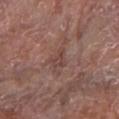Q: What is the lesion's diameter?
A: ~2.5 mm (longest diameter)
Q: How was the tile lit?
A: white-light
Q: Automated lesion metrics?
A: a lesion color around L≈43 a*≈20 b*≈23 in CIELAB and a lesion–skin lightness drop of about 7; border irregularity of about 5 on a 0–10 scale, a within-lesion color-variation index near 1/10, and radial color variation of about 0; a classifier nevus-likeness of about 0/100 and a detector confidence of about 90 out of 100 that the crop contains a lesion
Q: What are the patient's age and sex?
A: male, aged approximately 80
Q: How was this image acquired?
A: total-body-photography crop, ~15 mm field of view
Q: Lesion location?
A: the arm